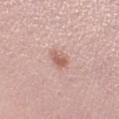follow-up — no biopsy performed (imaged during a skin exam); size — ~2.5 mm (longest diameter); anatomic site — the right lower leg; illumination — white-light; subject — female, roughly 25 years of age; imaging modality — ~15 mm crop, total-body skin-cancer survey.